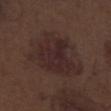Part of a total-body skin-imaging series; this lesion was reviewed on a skin check and was not flagged for biopsy. A close-up tile cropped from a whole-body skin photograph, about 15 mm across. The subject is a male aged 68 to 72. From the right lower leg.